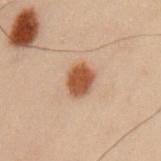Recorded during total-body skin imaging; not selected for excision or biopsy.
A male patient aged around 55.
Cropped from a total-body skin-imaging series; the visible field is about 15 mm.
From the left upper arm.